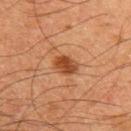{
  "biopsy_status": "not biopsied; imaged during a skin examination",
  "site": "upper back",
  "lesion_size": {
    "long_diameter_mm_approx": 3.0
  },
  "lighting": "cross-polarized",
  "automated_metrics": {
    "cielab_L": 38,
    "cielab_a": 23,
    "cielab_b": 33,
    "nevus_likeness_0_100": 95,
    "lesion_detection_confidence_0_100": 100
  },
  "patient": {
    "sex": "male",
    "age_approx": 65
  },
  "image": {
    "source": "total-body photography crop",
    "field_of_view_mm": 15
  }
}